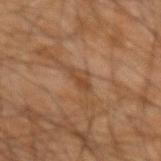Impression:
Imaged during a routine full-body skin examination; the lesion was not biopsied and no histopathology is available.
Acquisition and patient details:
Measured at roughly 3 mm in maximum diameter. Cropped from a whole-body photographic skin survey; the tile spans about 15 mm. The subject is a male aged 63–67. This is a cross-polarized tile. Located on the left forearm.